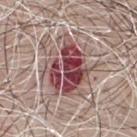| field | value |
|---|---|
| notes | imaged on a skin check; not biopsied |
| location | the chest |
| image-analysis metrics | an area of roughly 42 mm² and a shape eccentricity near 0.4 |
| imaging modality | total-body-photography crop, ~15 mm field of view |
| lesion size | ≈8.5 mm |
| patient | male, in their 70s |
| lighting | white-light illumination |